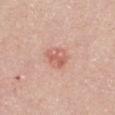On the chest.
This is a white-light tile.
The subject is a female in their mid-50s.
A lesion tile, about 15 mm wide, cut from a 3D total-body photograph.
About 3.5 mm across.